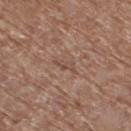No biopsy was performed on this lesion — it was imaged during a full skin examination and was not determined to be concerning. The lesion is on the right thigh. The total-body-photography lesion software estimated a lesion color around L≈48 a*≈18 b*≈26 in CIELAB and roughly 7 lightness units darker than nearby skin. The software also gave a border-irregularity index near 4/10 and a color-variation rating of about 0/10. The analysis additionally found a classifier nevus-likeness of about 0/100 and a detector confidence of about 85 out of 100 that the crop contains a lesion. Captured under white-light illumination. The recorded lesion diameter is about 3 mm. This image is a 15 mm lesion crop taken from a total-body photograph. The subject is a female roughly 75 years of age.